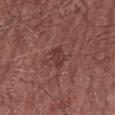Captured during whole-body skin photography for melanoma surveillance; the lesion was not biopsied. A male patient aged 73–77. The tile uses white-light illumination. The lesion is located on the right forearm. The recorded lesion diameter is about 2.5 mm. A region of skin cropped from a whole-body photographic capture, roughly 15 mm wide.